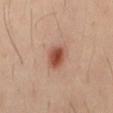{
  "site": "mid back",
  "patient": {
    "sex": "male",
    "age_approx": 40
  },
  "image": {
    "source": "total-body photography crop",
    "field_of_view_mm": 15
  }
}